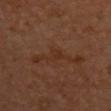Measured at roughly 2.5 mm in maximum diameter.
A female patient, in their mid- to late 30s.
Automated image analysis of the tile measured a footprint of about 3.5 mm², a shape eccentricity near 0.75, and a symmetry-axis asymmetry near 0.45. The analysis additionally found border irregularity of about 4.5 on a 0–10 scale, a color-variation rating of about 1/10, and radial color variation of about 0.5.
The lesion is on the chest.
This image is a 15 mm lesion crop taken from a total-body photograph.